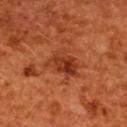Assessment:
No biopsy was performed on this lesion — it was imaged during a full skin examination and was not determined to be concerning.
Context:
A female patient, aged 48 to 52. The tile uses cross-polarized illumination. The lesion's longest dimension is about 4.5 mm. Located on the upper back. A lesion tile, about 15 mm wide, cut from a 3D total-body photograph.